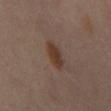biopsy status: catalogued during a skin exam; not biopsied
patient: female, roughly 50 years of age
automated lesion analysis: an area of roughly 6 mm², an eccentricity of roughly 0.85, and two-axis asymmetry of about 0.15; an average lesion color of about L≈34 a*≈16 b*≈24 (CIELAB), about 8 CIELAB-L* units darker than the surrounding skin, and a normalized lesion–skin contrast near 8.5; peripheral color asymmetry of about 1; a detector confidence of about 100 out of 100 that the crop contains a lesion
illumination: cross-polarized illumination
image: ~15 mm tile from a whole-body skin photo
body site: the front of the torso